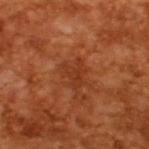| key | value |
|---|---|
| notes | catalogued during a skin exam; not biopsied |